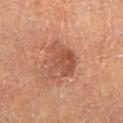Q: Was a biopsy performed?
A: total-body-photography surveillance lesion; no biopsy
Q: What is the lesion's diameter?
A: about 4.5 mm
Q: Lesion location?
A: the left lower leg
Q: What did automated image analysis measure?
A: an outline eccentricity of about 0.6 (0 = round, 1 = elongated) and a symmetry-axis asymmetry near 0.35; a lesion-to-skin contrast of about 6.5 (normalized; higher = more distinct); a border-irregularity rating of about 4/10, a color-variation rating of about 5/10, and peripheral color asymmetry of about 1.5
Q: What kind of image is this?
A: total-body-photography crop, ~15 mm field of view
Q: Who is the patient?
A: male, aged 83 or over